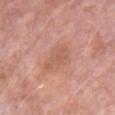Findings:
* workup — total-body-photography surveillance lesion; no biopsy